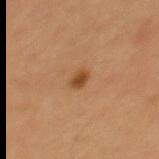<case>
  <biopsy_status>not biopsied; imaged during a skin examination</biopsy_status>
  <site>mid back</site>
  <image>
    <source>total-body photography crop</source>
    <field_of_view_mm>15</field_of_view_mm>
  </image>
  <automated_metrics>
    <cielab_L>45</cielab_L>
    <cielab_a>22</cielab_a>
    <cielab_b>36</cielab_b>
    <vs_skin_darker_L>10.0</vs_skin_darker_L>
    <vs_skin_contrast_norm>8.0</vs_skin_contrast_norm>
  </automated_metrics>
  <patient>
    <sex>male</sex>
    <age_approx>65</age_approx>
  </patient>
  <lighting>cross-polarized</lighting>
</case>